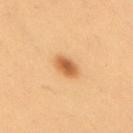<record>
<biopsy_status>not biopsied; imaged during a skin examination</biopsy_status>
<lighting>cross-polarized</lighting>
<lesion_size>
  <long_diameter_mm_approx>3.0</long_diameter_mm_approx>
</lesion_size>
<site>upper back</site>
<automated_metrics>
  <cielab_L>59</cielab_L>
  <cielab_a>23</cielab_a>
  <cielab_b>42</cielab_b>
  <vs_skin_darker_L>13.0</vs_skin_darker_L>
  <border_irregularity_0_10>1.5</border_irregularity_0_10>
  <color_variation_0_10>3.0</color_variation_0_10>
</automated_metrics>
<image>
  <source>total-body photography crop</source>
  <field_of_view_mm>15</field_of_view_mm>
</image>
<patient>
  <sex>male</sex>
  <age_approx>55</age_approx>
</patient>
</record>